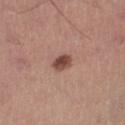| feature | finding |
|---|---|
| location | the right lower leg |
| tile lighting | white-light |
| image | 15 mm crop, total-body photography |
| lesion diameter | ~2.5 mm (longest diameter) |
| TBP lesion metrics | a lesion color around L≈46 a*≈23 b*≈25 in CIELAB, a lesion–skin lightness drop of about 14, and a normalized border contrast of about 10.5; a border-irregularity rating of about 2.5/10, a within-lesion color-variation index near 3.5/10, and a peripheral color-asymmetry measure near 1.5 |
| patient | male, aged 53 to 57 |